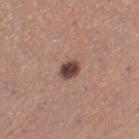Captured during whole-body skin photography for melanoma surveillance; the lesion was not biopsied. The patient is a female roughly 30 years of age. A lesion tile, about 15 mm wide, cut from a 3D total-body photograph. On the leg.